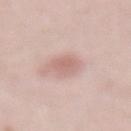Clinical impression: Recorded during total-body skin imaging; not selected for excision or biopsy. Image and clinical context: A female subject aged around 40. An algorithmic analysis of the crop reported a lesion area of about 4.5 mm², a shape eccentricity near 0.6, and a shape-asymmetry score of about 0.15 (0 = symmetric). This is a white-light tile. The lesion is located on the abdomen. A region of skin cropped from a whole-body photographic capture, roughly 15 mm wide. About 2.5 mm across.